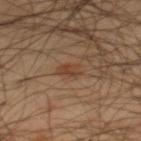Imaged during a routine full-body skin examination; the lesion was not biopsied and no histopathology is available. This is a cross-polarized tile. From the right lower leg. This image is a 15 mm lesion crop taken from a total-body photograph. Automated tile analysis of the lesion measured a classifier nevus-likeness of about 75/100 and lesion-presence confidence of about 100/100. The subject is a male in their mid-40s.